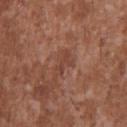Captured during whole-body skin photography for melanoma surveillance; the lesion was not biopsied. The recorded lesion diameter is about 2.5 mm. Imaged with white-light lighting. The patient is a male in their mid- to late 40s. Cropped from a total-body skin-imaging series; the visible field is about 15 mm. On the upper back.